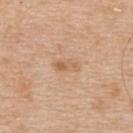{
  "biopsy_status": "not biopsied; imaged during a skin examination",
  "patient": {
    "sex": "male",
    "age_approx": 60
  },
  "lighting": "white-light",
  "lesion_size": {
    "long_diameter_mm_approx": 3.0
  },
  "site": "upper back",
  "image": {
    "source": "total-body photography crop",
    "field_of_view_mm": 15
  }
}